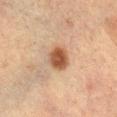Assessment: The lesion was tiled from a total-body skin photograph and was not biopsied. Image and clinical context: The lesion's longest dimension is about 3 mm. Automated tile analysis of the lesion measured a lesion color around L≈42 a*≈19 b*≈29 in CIELAB, roughly 13 lightness units darker than nearby skin, and a lesion-to-skin contrast of about 10 (normalized; higher = more distinct). The software also gave a border-irregularity rating of about 1.5/10, internal color variation of about 3 on a 0–10 scale, and radial color variation of about 1. A female subject aged around 55. Imaged with cross-polarized lighting. The lesion is on the right lower leg. A roughly 15 mm field-of-view crop from a total-body skin photograph.